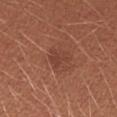biopsy status = imaged on a skin check; not biopsied
imaging modality = total-body-photography crop, ~15 mm field of view
TBP lesion metrics = a footprint of about 4 mm², an eccentricity of roughly 0.7, and a symmetry-axis asymmetry near 0.45; an average lesion color of about L≈41 a*≈24 b*≈28 (CIELAB), a lesion–skin lightness drop of about 6, and a normalized lesion–skin contrast near 5
illumination = white-light illumination
anatomic site = the right forearm
subject = female, roughly 25 years of age